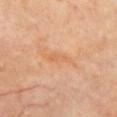follow-up: no biopsy performed (imaged during a skin exam) | subject: female, aged approximately 65 | site: the front of the torso | imaging modality: ~15 mm crop, total-body skin-cancer survey | diameter: ~3.5 mm (longest diameter) | automated metrics: an average lesion color of about L≈62 a*≈24 b*≈38 (CIELAB), a lesion–skin lightness drop of about 6, and a normalized border contrast of about 5; a border-irregularity rating of about 4.5/10, internal color variation of about 0.5 on a 0–10 scale, and a peripheral color-asymmetry measure near 0; a nevus-likeness score of about 0/100.